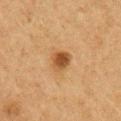Case summary:
* patient — male, about 75 years old
* diameter — ≈3 mm
* illumination — cross-polarized
* image source — total-body-photography crop, ~15 mm field of view
* TBP lesion metrics — an automated nevus-likeness rating near 95 out of 100 and a detector confidence of about 100 out of 100 that the crop contains a lesion
* body site — the chest